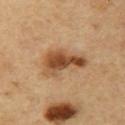Clinical impression:
Part of a total-body skin-imaging series; this lesion was reviewed on a skin check and was not flagged for biopsy.
Acquisition and patient details:
Automated image analysis of the tile measured an average lesion color of about L≈48 a*≈20 b*≈35 (CIELAB), a lesion–skin lightness drop of about 13, and a normalized border contrast of about 9.5. The software also gave a color-variation rating of about 6.5/10 and a peripheral color-asymmetry measure near 2.5. A 15 mm close-up tile from a total-body photography series done for melanoma screening. A male subject, aged approximately 55. The tile uses cross-polarized illumination. On the right upper arm.